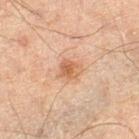The lesion was tiled from a total-body skin photograph and was not biopsied.
This is a cross-polarized tile.
The subject is a male about 65 years old.
The lesion is located on the left thigh.
Measured at roughly 2.5 mm in maximum diameter.
A 15 mm close-up extracted from a 3D total-body photography capture.